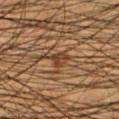<tbp_lesion>
<biopsy_status>not biopsied; imaged during a skin examination</biopsy_status>
<automated_metrics>
  <area_mm2_approx>3.5</area_mm2_approx>
  <border_irregularity_0_10>3.5</border_irregularity_0_10>
  <color_variation_0_10>3.0</color_variation_0_10>
  <peripheral_color_asymmetry>1.0</peripheral_color_asymmetry>
  <lesion_detection_confidence_0_100>75</lesion_detection_confidence_0_100>
</automated_metrics>
<patient>
  <sex>male</sex>
  <age_approx>45</age_approx>
</patient>
<site>left lower leg</site>
<image>
  <source>total-body photography crop</source>
  <field_of_view_mm>15</field_of_view_mm>
</image>
<lesion_size>
  <long_diameter_mm_approx>2.5</long_diameter_mm_approx>
</lesion_size>
<lighting>cross-polarized</lighting>
</tbp_lesion>